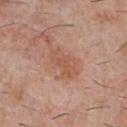follow-up: no biopsy performed (imaged during a skin exam)
subject: male, aged 28 to 32
diameter: ≈4.5 mm
anatomic site: the chest
acquisition: ~15 mm crop, total-body skin-cancer survey
automated metrics: an outline eccentricity of about 0.8 (0 = round, 1 = elongated) and a shape-asymmetry score of about 0.25 (0 = symmetric); a lesion color around L≈55 a*≈23 b*≈31 in CIELAB, about 7 CIELAB-L* units darker than the surrounding skin, and a normalized lesion–skin contrast near 5.5; an automated nevus-likeness rating near 10 out of 100 and lesion-presence confidence of about 100/100
tile lighting: white-light